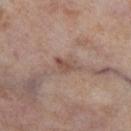<record>
  <biopsy_status>not biopsied; imaged during a skin examination</biopsy_status>
</record>